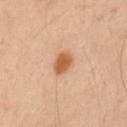Impression: This lesion was catalogued during total-body skin photography and was not selected for biopsy. Background: A male patient about 40 years old. The tile uses cross-polarized illumination. Cropped from a total-body skin-imaging series; the visible field is about 15 mm. Longest diameter approximately 3 mm. From the left upper arm.